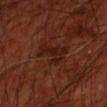biopsy_status: not biopsied; imaged during a skin examination
site: left forearm
lighting: cross-polarized
lesion_size:
  long_diameter_mm_approx: 4.5
patient:
  sex: male
  age_approx: 70
image:
  source: total-body photography crop
  field_of_view_mm: 15